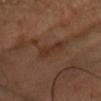On the head or neck. Automated image analysis of the tile measured an average lesion color of about L≈30 a*≈16 b*≈26 (CIELAB), a lesion–skin lightness drop of about 6, and a normalized lesion–skin contrast near 6.5. It also reported a classifier nevus-likeness of about 0/100. A male subject roughly 65 years of age. Captured under cross-polarized illumination. Approximately 5 mm at its widest. Cropped from a whole-body photographic skin survey; the tile spans about 15 mm.